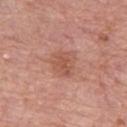Imaged during a routine full-body skin examination; the lesion was not biopsied and no histopathology is available. A roughly 15 mm field-of-view crop from a total-body skin photograph. Approximately 3.5 mm at its widest. Located on the left thigh. Imaged with white-light lighting. A female patient approximately 55 years of age.